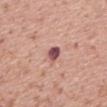{"biopsy_status": "not biopsied; imaged during a skin examination", "image": {"source": "total-body photography crop", "field_of_view_mm": 15}, "site": "mid back", "lesion_size": {"long_diameter_mm_approx": 2.5}, "patient": {"sex": "male", "age_approx": 70}, "lighting": "white-light"}